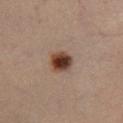Part of a total-body skin-imaging series; this lesion was reviewed on a skin check and was not flagged for biopsy. Cropped from a whole-body photographic skin survey; the tile spans about 15 mm. Approximately 2.5 mm at its widest. The subject is a male aged approximately 65. Located on the leg. The tile uses cross-polarized illumination.